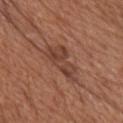The lesion was photographed on a routine skin check and not biopsied; there is no pathology result. A 15 mm close-up tile from a total-body photography series done for melanoma screening. A female subject in their mid- to late 70s. From the chest.